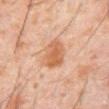biopsy status: imaged on a skin check; not biopsied
patient: male, in their 60s
acquisition: ~15 mm crop, total-body skin-cancer survey
lesion diameter: about 4 mm
tile lighting: cross-polarized
anatomic site: the abdomen